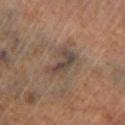Captured during whole-body skin photography for melanoma surveillance; the lesion was not biopsied. A 15 mm crop from a total-body photograph taken for skin-cancer surveillance. A female subject, aged approximately 65. On the left lower leg.